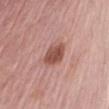Imaged during a routine full-body skin examination; the lesion was not biopsied and no histopathology is available.
The patient is a male in their mid-70s.
The recorded lesion diameter is about 3.5 mm.
The lesion is on the left upper arm.
A region of skin cropped from a whole-body photographic capture, roughly 15 mm wide.
The tile uses white-light illumination.